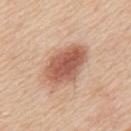The lesion was photographed on a routine skin check and not biopsied; there is no pathology result.
A 15 mm close-up extracted from a 3D total-body photography capture.
The lesion is located on the upper back.
About 6 mm across.
A male patient, about 60 years old.